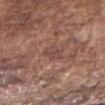{"biopsy_status": "not biopsied; imaged during a skin examination", "lesion_size": {"long_diameter_mm_approx": 2.5}, "image": {"source": "total-body photography crop", "field_of_view_mm": 15}, "site": "right forearm", "automated_metrics": {"area_mm2_approx": 4.0, "eccentricity": 0.75, "shape_asymmetry": 0.25, "border_irregularity_0_10": 2.5, "color_variation_0_10": 3.0, "nevus_likeness_0_100": 0, "lesion_detection_confidence_0_100": 85}, "patient": {"sex": "male", "age_approx": 75}}